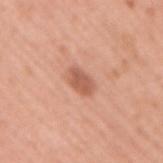<lesion>
  <biopsy_status>not biopsied; imaged during a skin examination</biopsy_status>
  <image>
    <source>total-body photography crop</source>
    <field_of_view_mm>15</field_of_view_mm>
  </image>
  <site>upper back</site>
  <patient>
    <sex>male</sex>
    <age_approx>45</age_approx>
  </patient>
</lesion>